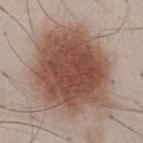<tbp_lesion>
  <biopsy_status>not biopsied; imaged during a skin examination</biopsy_status>
  <site>front of the torso</site>
  <patient>
    <sex>male</sex>
    <age_approx>50</age_approx>
  </patient>
  <lesion_size>
    <long_diameter_mm_approx>10.0</long_diameter_mm_approx>
  </lesion_size>
  <lighting>white-light</lighting>
  <image>
    <source>total-body photography crop</source>
    <field_of_view_mm>15</field_of_view_mm>
  </image>
  <automated_metrics>
    <cielab_L>50</cielab_L>
    <cielab_a>19</cielab_a>
    <cielab_b>26</cielab_b>
    <vs_skin_contrast_norm>10.5</vs_skin_contrast_norm>
    <border_irregularity_0_10>2.5</border_irregularity_0_10>
    <color_variation_0_10>5.0</color_variation_0_10>
  </automated_metrics>
</tbp_lesion>